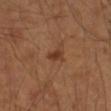{"biopsy_status": "not biopsied; imaged during a skin examination", "image": {"source": "total-body photography crop", "field_of_view_mm": 15}, "site": "right forearm", "lesion_size": {"long_diameter_mm_approx": 2.5}, "patient": {"sex": "male", "age_approx": 45}}